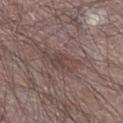workup: no biopsy performed (imaged during a skin exam) | acquisition: 15 mm crop, total-body photography | subject: male, aged 63–67 | size: ~5 mm (longest diameter) | automated lesion analysis: an average lesion color of about L≈43 a*≈16 b*≈18 (CIELAB), roughly 7 lightness units darker than nearby skin, and a normalized lesion–skin contrast near 5.5; a border-irregularity index near 4.5/10, internal color variation of about 3 on a 0–10 scale, and peripheral color asymmetry of about 1; an automated nevus-likeness rating near 0 out of 100 and lesion-presence confidence of about 65/100 | body site: the leg | lighting: white-light illumination.